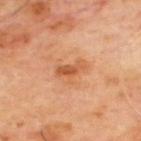Assessment: The lesion was photographed on a routine skin check and not biopsied; there is no pathology result. Background: The subject is a male aged 63 to 67. Imaged with cross-polarized lighting. Longest diameter approximately 3.5 mm. From the upper back. A 15 mm crop from a total-body photograph taken for skin-cancer surveillance.